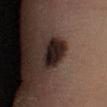lesion diameter: ≈4.5 mm
location: the left lower leg
acquisition: total-body-photography crop, ~15 mm field of view
patient: male, about 35 years old